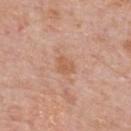Q: Was a biopsy performed?
A: no biopsy performed (imaged during a skin exam)
Q: Who is the patient?
A: male, about 75 years old
Q: What is the anatomic site?
A: the upper back
Q: What did automated image analysis measure?
A: an area of roughly 4 mm², a shape eccentricity near 0.65, and two-axis asymmetry of about 0.35; a border-irregularity rating of about 3/10, internal color variation of about 1.5 on a 0–10 scale, and radial color variation of about 0.5; a nevus-likeness score of about 0/100
Q: What is the imaging modality?
A: ~15 mm crop, total-body skin-cancer survey
Q: Lesion size?
A: ~2.5 mm (longest diameter)
Q: How was the tile lit?
A: white-light